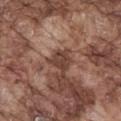Q: Was this lesion biopsied?
A: imaged on a skin check; not biopsied
Q: What lighting was used for the tile?
A: white-light
Q: What is the imaging modality?
A: total-body-photography crop, ~15 mm field of view
Q: Patient demographics?
A: male, aged around 75
Q: What is the anatomic site?
A: the mid back